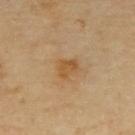Clinical impression:
Captured during whole-body skin photography for melanoma surveillance; the lesion was not biopsied.
Clinical summary:
Measured at roughly 2.5 mm in maximum diameter. Captured under cross-polarized illumination. Located on the upper back. A 15 mm close-up tile from a total-body photography series done for melanoma screening. A female subject aged 68–72. An algorithmic analysis of the crop reported a footprint of about 4 mm², an outline eccentricity of about 0.55 (0 = round, 1 = elongated), and a shape-asymmetry score of about 0.4 (0 = symmetric).